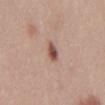| feature | finding |
|---|---|
| notes | no biopsy performed (imaged during a skin exam) |
| location | the mid back |
| subject | male, approximately 25 years of age |
| illumination | white-light |
| size | ≈3 mm |
| image | ~15 mm tile from a whole-body skin photo |
| TBP lesion metrics | an area of roughly 4 mm², an eccentricity of roughly 0.85, and two-axis asymmetry of about 0.25; an automated nevus-likeness rating near 100 out of 100 and lesion-presence confidence of about 100/100 |